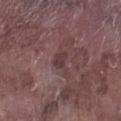| feature | finding |
|---|---|
| patient | male, aged 73–77 |
| lesion diameter | about 2.5 mm |
| lighting | white-light illumination |
| image | ~15 mm tile from a whole-body skin photo |
| site | the leg |